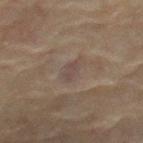The lesion was photographed on a routine skin check and not biopsied; there is no pathology result.